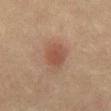• notes — total-body-photography surveillance lesion; no biopsy
• image source — 15 mm crop, total-body photography
• body site — the abdomen
• subject — female, aged around 55
• lesion size — ≈3.5 mm